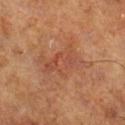Assessment: The lesion was photographed on a routine skin check and not biopsied; there is no pathology result. Background: From the leg. A 15 mm crop from a total-body photograph taken for skin-cancer surveillance. The recorded lesion diameter is about 6.5 mm. A male patient aged 68 to 72. Imaged with cross-polarized lighting.